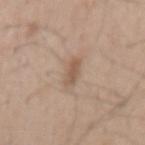biopsy_status: not biopsied; imaged during a skin examination
automated_metrics:
  border_irregularity_0_10: 2.0
  peripheral_color_asymmetry: 0.5
patient:
  sex: male
  age_approx: 50
site: back
lesion_size:
  long_diameter_mm_approx: 3.0
image:
  source: total-body photography crop
  field_of_view_mm: 15
lighting: white-light